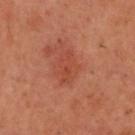Q: Is there a histopathology result?
A: catalogued during a skin exam; not biopsied
Q: Where on the body is the lesion?
A: the head or neck
Q: How large is the lesion?
A: ~3.5 mm (longest diameter)
Q: Who is the patient?
A: male, roughly 55 years of age
Q: How was this image acquired?
A: ~15 mm crop, total-body skin-cancer survey
Q: What did automated image analysis measure?
A: an average lesion color of about L≈37 a*≈25 b*≈28 (CIELAB), a lesion–skin lightness drop of about 5, and a lesion-to-skin contrast of about 4.5 (normalized; higher = more distinct); a border-irregularity rating of about 2/10, a color-variation rating of about 2.5/10, and peripheral color asymmetry of about 1
Q: What lighting was used for the tile?
A: cross-polarized